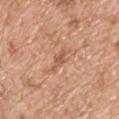- follow-up: catalogued during a skin exam; not biopsied
- acquisition: ~15 mm crop, total-body skin-cancer survey
- location: the upper back
- patient: male, in their mid- to late 70s